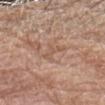Impression: Captured during whole-body skin photography for melanoma surveillance; the lesion was not biopsied. Background: From the left forearm. A 15 mm close-up extracted from a 3D total-body photography capture. An algorithmic analysis of the crop reported a mean CIELAB color near L≈55 a*≈19 b*≈29, roughly 6 lightness units darker than nearby skin, and a lesion-to-skin contrast of about 4.5 (normalized; higher = more distinct). The software also gave a border-irregularity rating of about 5.5/10 and a within-lesion color-variation index near 0/10. The analysis additionally found a classifier nevus-likeness of about 0/100 and a lesion-detection confidence of about 90/100. A male subject in their 80s. Captured under white-light illumination.